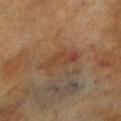Q: Was this lesion biopsied?
A: catalogued during a skin exam; not biopsied
Q: What is the imaging modality?
A: total-body-photography crop, ~15 mm field of view
Q: Lesion size?
A: about 5 mm
Q: Who is the patient?
A: male, aged 68–72
Q: Where on the body is the lesion?
A: the right lower leg